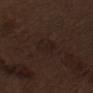Impression:
This lesion was catalogued during total-body skin photography and was not selected for biopsy.
Context:
A male patient in their 70s. From the left upper arm. A 15 mm close-up tile from a total-body photography series done for melanoma screening. Captured under white-light illumination.